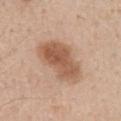The lesion was tiled from a total-body skin photograph and was not biopsied. About 6.5 mm across. A male subject aged around 60. On the back. A 15 mm close-up tile from a total-body photography series done for melanoma screening. The tile uses white-light illumination.